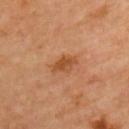notes: total-body-photography surveillance lesion; no biopsy
location: the arm
patient: female, about 50 years old
acquisition: 15 mm crop, total-body photography